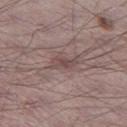Clinical impression:
Part of a total-body skin-imaging series; this lesion was reviewed on a skin check and was not flagged for biopsy.
Acquisition and patient details:
The lesion is located on the left thigh. A 15 mm close-up tile from a total-body photography series done for melanoma screening. The subject is a male aged 68 to 72.